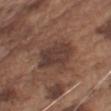{"biopsy_status": "not biopsied; imaged during a skin examination", "site": "left upper arm", "lighting": "white-light", "patient": {"sex": "male", "age_approx": 75}, "image": {"source": "total-body photography crop", "field_of_view_mm": 15}, "automated_metrics": {"area_mm2_approx": 13.0, "eccentricity": 0.85, "shape_asymmetry": 0.3, "border_irregularity_0_10": 3.5, "color_variation_0_10": 3.0, "peripheral_color_asymmetry": 1.0, "nevus_likeness_0_100": 5}, "lesion_size": {"long_diameter_mm_approx": 5.5}}